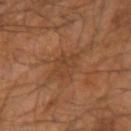Part of a total-body skin-imaging series; this lesion was reviewed on a skin check and was not flagged for biopsy. A 15 mm close-up tile from a total-body photography series done for melanoma screening. A male patient aged around 65. Imaged with cross-polarized lighting. Located on the arm.